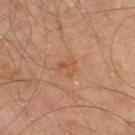{"biopsy_status": "not biopsied; imaged during a skin examination", "site": "right thigh", "lesion_size": {"long_diameter_mm_approx": 2.5}, "lighting": "cross-polarized", "image": {"source": "total-body photography crop", "field_of_view_mm": 15}, "patient": {"sex": "male", "age_approx": 60}}